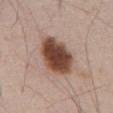| key | value |
|---|---|
| biopsy status | imaged on a skin check; not biopsied |
| patient | male, aged around 55 |
| site | the abdomen |
| imaging modality | total-body-photography crop, ~15 mm field of view |
| size | about 5.5 mm |